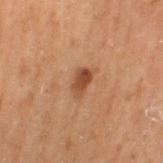Part of a total-body skin-imaging series; this lesion was reviewed on a skin check and was not flagged for biopsy.
A male patient, aged 68 to 72.
Automated tile analysis of the lesion measured a mean CIELAB color near L≈35 a*≈19 b*≈28, about 9 CIELAB-L* units darker than the surrounding skin, and a normalized border contrast of about 8.5.
A 15 mm close-up tile from a total-body photography series done for melanoma screening.
This is a cross-polarized tile.
On the left thigh.
The lesion's longest dimension is about 3 mm.